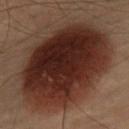follow-up: imaged on a skin check; not biopsied | automated metrics: an outline eccentricity of about 0.6 (0 = round, 1 = elongated) and a shape-asymmetry score of about 0.1 (0 = symmetric); a border-irregularity index near 1.5/10, a color-variation rating of about 6/10, and a peripheral color-asymmetry measure near 2 | lesion diameter: ≈11 mm | illumination: cross-polarized illumination | image source: total-body-photography crop, ~15 mm field of view | subject: male, aged around 55 | location: the chest.